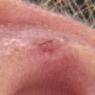Impression: Part of a total-body skin-imaging series; this lesion was reviewed on a skin check and was not flagged for biopsy. Background: The recorded lesion diameter is about 2.5 mm. This is a white-light tile. The lesion is located on the head or neck. The patient is a female about 40 years old. A 15 mm close-up extracted from a 3D total-body photography capture.